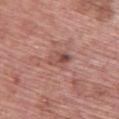Assessment: The lesion was tiled from a total-body skin photograph and was not biopsied. Clinical summary: This is a white-light tile. Located on the back. The subject is a male roughly 60 years of age. A 15 mm close-up tile from a total-body photography series done for melanoma screening. The lesion's longest dimension is about 3 mm.